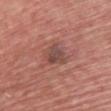{"patient": {"sex": "male", "age_approx": 60}, "image": {"source": "total-body photography crop", "field_of_view_mm": 15}, "site": "upper back"}